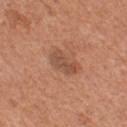Q: Is there a histopathology result?
A: total-body-photography surveillance lesion; no biopsy
Q: What lighting was used for the tile?
A: white-light
Q: Lesion size?
A: about 3.5 mm
Q: Lesion location?
A: the chest
Q: What are the patient's age and sex?
A: male, aged 63 to 67
Q: What is the imaging modality?
A: total-body-photography crop, ~15 mm field of view